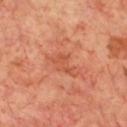Notes:
– workup: total-body-photography surveillance lesion; no biopsy
– size: ~3.5 mm (longest diameter)
– location: the chest
– subject: male, about 70 years old
– lighting: cross-polarized
– image: ~15 mm tile from a whole-body skin photo
– automated metrics: a mean CIELAB color near L≈52 a*≈30 b*≈35 and roughly 6 lightness units darker than nearby skin; a border-irregularity rating of about 5.5/10, a color-variation rating of about 2/10, and a peripheral color-asymmetry measure near 0.5